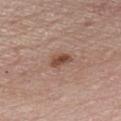{"automated_metrics": {"border_irregularity_0_10": 3.0, "color_variation_0_10": 3.0, "peripheral_color_asymmetry": 1.0, "nevus_likeness_0_100": 85}, "image": {"source": "total-body photography crop", "field_of_view_mm": 15}, "patient": {"sex": "male", "age_approx": 60}, "site": "front of the torso"}